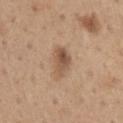<record>
  <biopsy_status>not biopsied; imaged during a skin examination</biopsy_status>
  <patient>
    <sex>male</sex>
    <age_approx>65</age_approx>
  </patient>
  <lighting>white-light</lighting>
  <image>
    <source>total-body photography crop</source>
    <field_of_view_mm>15</field_of_view_mm>
  </image>
  <site>front of the torso</site>
  <automated_metrics>
    <cielab_L>54</cielab_L>
    <cielab_a>18</cielab_a>
    <cielab_b>31</cielab_b>
    <vs_skin_darker_L>11.0</vs_skin_darker_L>
    <vs_skin_contrast_norm>7.5</vs_skin_contrast_norm>
    <border_irregularity_0_10>2.5</border_irregularity_0_10>
    <color_variation_0_10>6.0</color_variation_0_10>
    <peripheral_color_asymmetry>2.5</peripheral_color_asymmetry>
    <nevus_likeness_0_100>80</nevus_likeness_0_100>
    <lesion_detection_confidence_0_100>100</lesion_detection_confidence_0_100>
  </automated_metrics>
  <lesion_size>
    <long_diameter_mm_approx>4.0</long_diameter_mm_approx>
  </lesion_size>
</record>